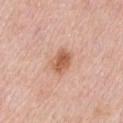Imaged during a routine full-body skin examination; the lesion was not biopsied and no histopathology is available. Measured at roughly 3 mm in maximum diameter. A close-up tile cropped from a whole-body skin photograph, about 15 mm across. On the chest. The patient is a male in their 80s. Captured under white-light illumination.